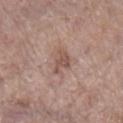Q: What kind of image is this?
A: ~15 mm tile from a whole-body skin photo
Q: What lighting was used for the tile?
A: white-light
Q: Patient demographics?
A: female, aged 83 or over
Q: Where on the body is the lesion?
A: the left lower leg
Q: What is the lesion's diameter?
A: ≈3.5 mm
Q: What did automated image analysis measure?
A: a border-irregularity rating of about 4.5/10 and peripheral color asymmetry of about 0.5; a nevus-likeness score of about 0/100 and a lesion-detection confidence of about 100/100